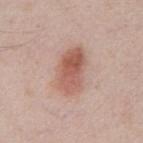Captured during whole-body skin photography for melanoma surveillance; the lesion was not biopsied. A 15 mm close-up extracted from a 3D total-body photography capture. The total-body-photography lesion software estimated a footprint of about 14 mm² and an outline eccentricity of about 0.85 (0 = round, 1 = elongated). It also reported a within-lesion color-variation index near 6/10 and radial color variation of about 2. The lesion's longest dimension is about 5.5 mm. A male patient about 50 years old. The lesion is located on the front of the torso. Imaged with white-light lighting.